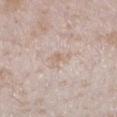From the left lower leg. The total-body-photography lesion software estimated a mean CIELAB color near L≈66 a*≈14 b*≈26, roughly 6 lightness units darker than nearby skin, and a normalized border contrast of about 5. It also reported a nevus-likeness score of about 0/100 and a lesion-detection confidence of about 65/100. A female patient approximately 25 years of age. A region of skin cropped from a whole-body photographic capture, roughly 15 mm wide.